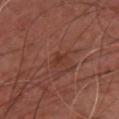A male patient, aged approximately 45. The lesion is located on the chest. Automated image analysis of the tile measured an average lesion color of about L≈32 a*≈22 b*≈26 (CIELAB), about 5 CIELAB-L* units darker than the surrounding skin, and a normalized border contrast of about 5.5. Cropped from a whole-body photographic skin survey; the tile spans about 15 mm. The lesion's longest dimension is about 2 mm. This is a cross-polarized tile.